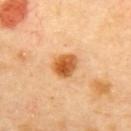Notes:
– notes — catalogued during a skin exam; not biopsied
– illumination — cross-polarized
– imaging modality — ~15 mm crop, total-body skin-cancer survey
– patient — female, aged approximately 60
– automated lesion analysis — an average lesion color of about L≈61 a*≈27 b*≈47 (CIELAB) and a lesion-to-skin contrast of about 10.5 (normalized; higher = more distinct); lesion-presence confidence of about 100/100
– lesion size — about 3.5 mm
– body site — the upper back